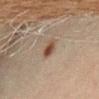The lesion was tiled from a total-body skin photograph and was not biopsied.
Located on the lower back.
Measured at roughly 2.5 mm in maximum diameter.
A male patient, aged 68–72.
A close-up tile cropped from a whole-body skin photograph, about 15 mm across.
This is a cross-polarized tile.